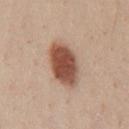Assessment: The lesion was photographed on a routine skin check and not biopsied; there is no pathology result. Context: Automated tile analysis of the lesion measured a lesion area of about 14 mm² and a shape eccentricity near 0.8. And it measured an average lesion color of about L≈51 a*≈22 b*≈29 (CIELAB), about 17 CIELAB-L* units darker than the surrounding skin, and a normalized border contrast of about 11.5. The software also gave a classifier nevus-likeness of about 100/100 and a detector confidence of about 100 out of 100 that the crop contains a lesion. A male subject, approximately 40 years of age. The recorded lesion diameter is about 5.5 mm. Located on the chest. Cropped from a total-body skin-imaging series; the visible field is about 15 mm.